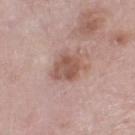The lesion was photographed on a routine skin check and not biopsied; there is no pathology result. The tile uses white-light illumination. The subject is a male aged 78–82. A roughly 15 mm field-of-view crop from a total-body skin photograph. From the right lower leg.